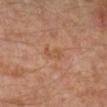This lesion was catalogued during total-body skin photography and was not selected for biopsy. Automated image analysis of the tile measured a lesion area of about 3.5 mm², an outline eccentricity of about 0.85 (0 = round, 1 = elongated), and a symmetry-axis asymmetry near 0.25. This image is a 15 mm lesion crop taken from a total-body photograph. On the leg. Approximately 3 mm at its widest. A male subject about 30 years old.